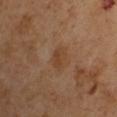Recorded during total-body skin imaging; not selected for excision or biopsy.
The lesion's longest dimension is about 3 mm.
A male subject approximately 65 years of age.
A close-up tile cropped from a whole-body skin photograph, about 15 mm across.
Imaged with cross-polarized lighting.